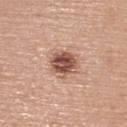biopsy status: total-body-photography surveillance lesion; no biopsy
lesion diameter: ~3.5 mm (longest diameter)
subject: female, aged approximately 65
anatomic site: the upper back
image-analysis metrics: an average lesion color of about L≈52 a*≈22 b*≈28 (CIELAB), roughly 16 lightness units darker than nearby skin, and a normalized lesion–skin contrast near 11; a within-lesion color-variation index near 5.5/10 and radial color variation of about 2; an automated nevus-likeness rating near 70 out of 100 and a lesion-detection confidence of about 100/100
acquisition: total-body-photography crop, ~15 mm field of view
illumination: white-light